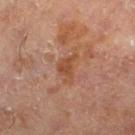- follow-up · catalogued during a skin exam; not biopsied